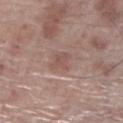notes: catalogued during a skin exam; not biopsied | image source: total-body-photography crop, ~15 mm field of view | patient: male, about 70 years old | site: the right lower leg | TBP lesion metrics: an average lesion color of about L≈52 a*≈19 b*≈23 (CIELAB), about 7 CIELAB-L* units darker than the surrounding skin, and a lesion-to-skin contrast of about 5 (normalized; higher = more distinct) | tile lighting: white-light | lesion diameter: ~2.5 mm (longest diameter).